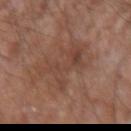Clinical impression: The lesion was photographed on a routine skin check and not biopsied; there is no pathology result. Context: Longest diameter approximately 6.5 mm. A male patient aged 53–57. From the right forearm. Captured under white-light illumination. Cropped from a total-body skin-imaging series; the visible field is about 15 mm.